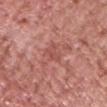The lesion was photographed on a routine skin check and not biopsied; there is no pathology result. Automated tile analysis of the lesion measured a footprint of about 4 mm², an eccentricity of roughly 0.7, and a symmetry-axis asymmetry near 0.35. The subject is a male aged 48 to 52. Cropped from a whole-body photographic skin survey; the tile spans about 15 mm. Approximately 2.5 mm at its widest. Located on the head or neck.